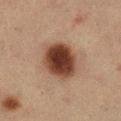Approximately 5 mm at its widest. Automated image analysis of the tile measured an eccentricity of roughly 0.6 and two-axis asymmetry of about 0.1. It also reported a border-irregularity index near 1/10, internal color variation of about 4 on a 0–10 scale, and peripheral color asymmetry of about 1. The analysis additionally found an automated nevus-likeness rating near 100 out of 100 and a detector confidence of about 100 out of 100 that the crop contains a lesion. This is a cross-polarized tile. The lesion is located on the right lower leg. A female patient, aged around 55. A 15 mm close-up extracted from a 3D total-body photography capture.